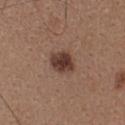The lesion was tiled from a total-body skin photograph and was not biopsied. The lesion-visualizer software estimated roughly 14 lightness units darker than nearby skin and a normalized border contrast of about 11.5. And it measured internal color variation of about 3 on a 0–10 scale and a peripheral color-asymmetry measure near 1. Captured under white-light illumination. A female subject, approximately 40 years of age. The lesion is on the head or neck. Longest diameter approximately 3 mm. This image is a 15 mm lesion crop taken from a total-body photograph.